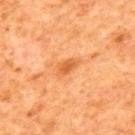The lesion was photographed on a routine skin check and not biopsied; there is no pathology result. On the upper back. Automated image analysis of the tile measured a lesion area of about 3.5 mm², a shape eccentricity near 0.8, and a shape-asymmetry score of about 0.25 (0 = symmetric). And it measured a nevus-likeness score of about 40/100. The recorded lesion diameter is about 2.5 mm. A 15 mm close-up extracted from a 3D total-body photography capture. Captured under cross-polarized illumination. A male patient aged around 80.